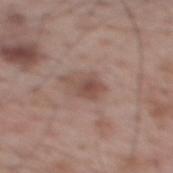This lesion was catalogued during total-body skin photography and was not selected for biopsy.
A lesion tile, about 15 mm wide, cut from a 3D total-body photograph.
A male patient about 70 years old.
Automated image analysis of the tile measured a footprint of about 5 mm² and a symmetry-axis asymmetry near 0.45. It also reported a mean CIELAB color near L≈48 a*≈19 b*≈23 and a lesion-to-skin contrast of about 7 (normalized; higher = more distinct). The analysis additionally found a within-lesion color-variation index near 2.5/10 and peripheral color asymmetry of about 1.
The lesion's longest dimension is about 4 mm.
Located on the upper back.
Imaged with white-light lighting.